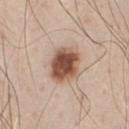Q: Was this lesion biopsied?
A: no biopsy performed (imaged during a skin exam)
Q: Lesion size?
A: ~4 mm (longest diameter)
Q: Who is the patient?
A: male, about 45 years old
Q: What is the imaging modality?
A: total-body-photography crop, ~15 mm field of view
Q: Lesion location?
A: the chest
Q: What lighting was used for the tile?
A: white-light illumination